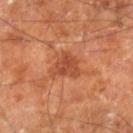Part of a total-body skin-imaging series; this lesion was reviewed on a skin check and was not flagged for biopsy.